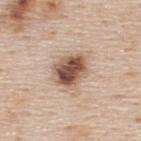follow-up: catalogued during a skin exam; not biopsied | acquisition: ~15 mm crop, total-body skin-cancer survey | lesion size: ~4.5 mm (longest diameter) | patient: male, approximately 45 years of age | body site: the upper back.